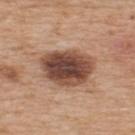* follow-up: catalogued during a skin exam; not biopsied
* subject: male, approximately 65 years of age
* diameter: ≈6 mm
* imaging modality: total-body-photography crop, ~15 mm field of view
* tile lighting: white-light
* body site: the upper back